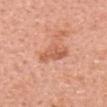Imaged during a routine full-body skin examination; the lesion was not biopsied and no histopathology is available.
Automated tile analysis of the lesion measured a footprint of about 7 mm² and an eccentricity of roughly 0.8. The software also gave about 9 CIELAB-L* units darker than the surrounding skin and a normalized border contrast of about 6.5. The software also gave border irregularity of about 4 on a 0–10 scale and peripheral color asymmetry of about 1.5. And it measured an automated nevus-likeness rating near 5 out of 100.
Located on the head or neck.
This is a white-light tile.
A female patient, aged 63–67.
Approximately 4 mm at its widest.
This image is a 15 mm lesion crop taken from a total-body photograph.